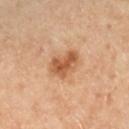Imaged during a routine full-body skin examination; the lesion was not biopsied and no histopathology is available. A 15 mm close-up extracted from a 3D total-body photography capture. Imaged with cross-polarized lighting. A male subject, aged 63–67. The total-body-photography lesion software estimated a footprint of about 8 mm². The software also gave a mean CIELAB color near L≈56 a*≈24 b*≈37, about 12 CIELAB-L* units darker than the surrounding skin, and a normalized border contrast of about 8.5. It also reported a border-irregularity rating of about 2.5/10, a color-variation rating of about 4/10, and peripheral color asymmetry of about 1.5. The lesion is located on the right lower leg.